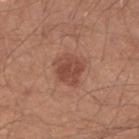Imaged during a routine full-body skin examination; the lesion was not biopsied and no histopathology is available.
On the left forearm.
Cropped from a total-body skin-imaging series; the visible field is about 15 mm.
A male subject, aged 28 to 32.